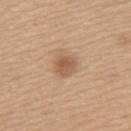<tbp_lesion>
  <patient>
    <sex>female</sex>
    <age_approx>65</age_approx>
  </patient>
  <site>back</site>
  <image>
    <source>total-body photography crop</source>
    <field_of_view_mm>15</field_of_view_mm>
  </image>
  <lighting>white-light</lighting>
</tbp_lesion>